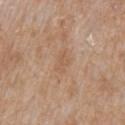Impression:
Part of a total-body skin-imaging series; this lesion was reviewed on a skin check and was not flagged for biopsy.
Image and clinical context:
Imaged with white-light lighting. This image is a 15 mm lesion crop taken from a total-body photograph. Located on the lower back. The recorded lesion diameter is about 2.5 mm. Automated tile analysis of the lesion measured a footprint of about 3.5 mm². It also reported a mean CIELAB color near L≈57 a*≈18 b*≈32, about 6 CIELAB-L* units darker than the surrounding skin, and a lesion-to-skin contrast of about 4.5 (normalized; higher = more distinct). The analysis additionally found a border-irregularity rating of about 3/10 and a within-lesion color-variation index near 1.5/10. And it measured an automated nevus-likeness rating near 0 out of 100 and lesion-presence confidence of about 100/100. The subject is a male aged 58–62.